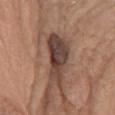Impression: Part of a total-body skin-imaging series; this lesion was reviewed on a skin check and was not flagged for biopsy. Background: A female patient aged 58–62. Cropped from a total-body skin-imaging series; the visible field is about 15 mm. On the chest. Imaged with white-light lighting. An algorithmic analysis of the crop reported a footprint of about 15 mm² and a shape-asymmetry score of about 0.35 (0 = symmetric).